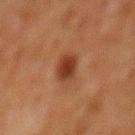notes — catalogued during a skin exam; not biopsied.